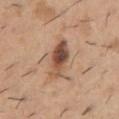A male patient approximately 40 years of age.
The tile uses white-light illumination.
Longest diameter approximately 5 mm.
Located on the chest.
A 15 mm close-up extracted from a 3D total-body photography capture.
An algorithmic analysis of the crop reported a lesion–skin lightness drop of about 15 and a lesion-to-skin contrast of about 10 (normalized; higher = more distinct).